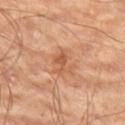imaging modality=total-body-photography crop, ~15 mm field of view | lesion size=about 3 mm | illumination=cross-polarized | subject=male, in their mid- to late 60s | anatomic site=the left thigh.